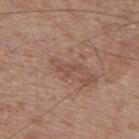biopsy status = imaged on a skin check; not biopsied
TBP lesion metrics = a mean CIELAB color near L≈49 a*≈19 b*≈25, roughly 7 lightness units darker than nearby skin, and a normalized lesion–skin contrast near 5; a within-lesion color-variation index near 0/10 and radial color variation of about 0
location = the upper back
subject = male, approximately 60 years of age
imaging modality = ~15 mm crop, total-body skin-cancer survey
illumination = white-light illumination
size = about 2.5 mm